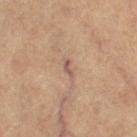Clinical impression: Imaged during a routine full-body skin examination; the lesion was not biopsied and no histopathology is available. Background: A 15 mm crop from a total-body photograph taken for skin-cancer surveillance. The lesion is located on the leg. A female patient, aged around 65.